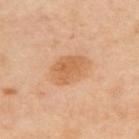Findings:
- biopsy status: total-body-photography surveillance lesion; no biopsy
- automated metrics: a lesion area of about 12 mm², an outline eccentricity of about 0.75 (0 = round, 1 = elongated), and a symmetry-axis asymmetry near 0.15; an average lesion color of about L≈64 a*≈23 b*≈40 (CIELAB) and a lesion–skin lightness drop of about 9; a detector confidence of about 100 out of 100 that the crop contains a lesion
- size: ~4.5 mm (longest diameter)
- lighting: cross-polarized illumination
- imaging modality: ~15 mm crop, total-body skin-cancer survey
- subject: female, aged approximately 40
- anatomic site: the upper back